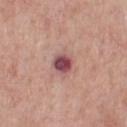Recorded during total-body skin imaging; not selected for excision or biopsy. A 15 mm close-up tile from a total-body photography series done for melanoma screening. About 2.5 mm across. A male patient about 55 years old. This is a white-light tile. The lesion is on the chest.